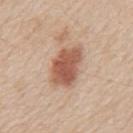This lesion was catalogued during total-body skin photography and was not selected for biopsy.
The lesion is on the mid back.
Automated image analysis of the tile measured a lesion color around L≈56 a*≈23 b*≈30 in CIELAB, a lesion–skin lightness drop of about 14, and a lesion-to-skin contrast of about 9.5 (normalized; higher = more distinct). And it measured a classifier nevus-likeness of about 100/100 and a lesion-detection confidence of about 100/100.
A close-up tile cropped from a whole-body skin photograph, about 15 mm across.
The patient is a male in their 60s.
This is a white-light tile.
About 5 mm across.